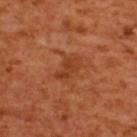This lesion was catalogued during total-body skin photography and was not selected for biopsy. Automated image analysis of the tile measured an outline eccentricity of about 0.85 (0 = round, 1 = elongated) and a symmetry-axis asymmetry near 0.35. The software also gave border irregularity of about 4 on a 0–10 scale, internal color variation of about 2.5 on a 0–10 scale, and radial color variation of about 1. On the upper back. Approximately 3.5 mm at its widest. A close-up tile cropped from a whole-body skin photograph, about 15 mm across. The patient is a female in their mid- to late 50s. This is a cross-polarized tile.